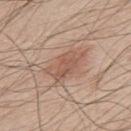Recorded during total-body skin imaging; not selected for excision or biopsy. A male patient approximately 50 years of age. Measured at roughly 5 mm in maximum diameter. A region of skin cropped from a whole-body photographic capture, roughly 15 mm wide. Located on the upper back. Captured under white-light illumination.